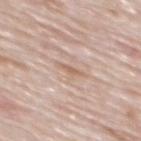Clinical impression:
Recorded during total-body skin imaging; not selected for excision or biopsy.
Background:
On the mid back. A male patient roughly 80 years of age. This is a white-light tile. A region of skin cropped from a whole-body photographic capture, roughly 15 mm wide.